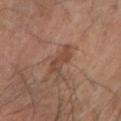Recorded during total-body skin imaging; not selected for excision or biopsy.
This is a cross-polarized tile.
An algorithmic analysis of the crop reported about 8 CIELAB-L* units darker than the surrounding skin and a normalized lesion–skin contrast near 6.5.
The lesion is on the right forearm.
The patient is a male in their mid-60s.
Measured at roughly 3.5 mm in maximum diameter.
A close-up tile cropped from a whole-body skin photograph, about 15 mm across.